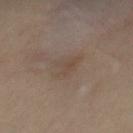automated metrics: a footprint of about 6.5 mm², an eccentricity of roughly 0.75, and a shape-asymmetry score of about 0.3 (0 = symmetric); a border-irregularity index near 3/10, a color-variation rating of about 2/10, and a peripheral color-asymmetry measure near 0.5
site: the mid back
image source: ~15 mm crop, total-body skin-cancer survey
size: ≈4 mm
patient: male, aged 33–37